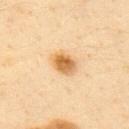The lesion's longest dimension is about 3 mm. Cropped from a whole-body photographic skin survey; the tile spans about 15 mm. Captured under cross-polarized illumination. Automated tile analysis of the lesion measured a shape eccentricity near 0.65 and a symmetry-axis asymmetry near 0.1. The analysis additionally found an average lesion color of about L≈59 a*≈18 b*≈40 (CIELAB), roughly 13 lightness units darker than nearby skin, and a normalized border contrast of about 9.5. A male subject, about 35 years old. From the back.